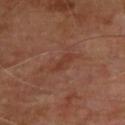{"biopsy_status": "not biopsied; imaged during a skin examination", "site": "upper back", "patient": {"sex": "male", "age_approx": 70}, "image": {"source": "total-body photography crop", "field_of_view_mm": 15}}